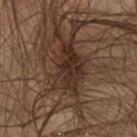workup: no biopsy performed (imaged during a skin exam) | image-analysis metrics: an area of roughly 14 mm², an outline eccentricity of about 0.8 (0 = round, 1 = elongated), and two-axis asymmetry of about 0.35; an average lesion color of about L≈23 a*≈13 b*≈19 (CIELAB), about 8 CIELAB-L* units darker than the surrounding skin, and a lesion-to-skin contrast of about 9.5 (normalized; higher = more distinct); a detector confidence of about 65 out of 100 that the crop contains a lesion | anatomic site: the front of the torso | acquisition: 15 mm crop, total-body photography | illumination: cross-polarized illumination | subject: male, about 50 years old | diameter: about 6 mm.